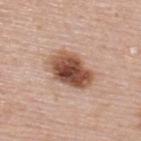Q: Was this lesion biopsied?
A: catalogued during a skin exam; not biopsied
Q: What is the imaging modality?
A: 15 mm crop, total-body photography
Q: What did automated image analysis measure?
A: a footprint of about 15 mm² and a shape eccentricity near 0.75; an average lesion color of about L≈51 a*≈22 b*≈29 (CIELAB), about 18 CIELAB-L* units darker than the surrounding skin, and a lesion-to-skin contrast of about 11.5 (normalized; higher = more distinct); a border-irregularity index near 2.5/10, internal color variation of about 8 on a 0–10 scale, and radial color variation of about 2.5
Q: What is the lesion's diameter?
A: ~5.5 mm (longest diameter)
Q: How was the tile lit?
A: white-light
Q: Lesion location?
A: the upper back
Q: Who is the patient?
A: female, aged approximately 50